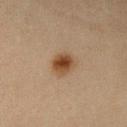Captured during whole-body skin photography for melanoma surveillance; the lesion was not biopsied. Cropped from a whole-body photographic skin survey; the tile spans about 15 mm. Approximately 2.5 mm at its widest. From the right forearm. A female subject, approximately 40 years of age.